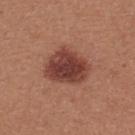Clinical impression:
The lesion was tiled from a total-body skin photograph and was not biopsied.